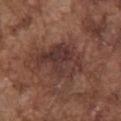The lesion was photographed on a routine skin check and not biopsied; there is no pathology result.
Captured under white-light illumination.
The subject is a male aged around 75.
The lesion is on the chest.
A region of skin cropped from a whole-body photographic capture, roughly 15 mm wide.
Automated image analysis of the tile measured a footprint of about 20 mm², an eccentricity of roughly 0.75, and two-axis asymmetry of about 0.3. The analysis additionally found a lesion color around L≈35 a*≈20 b*≈22 in CIELAB, about 8 CIELAB-L* units darker than the surrounding skin, and a normalized border contrast of about 8.5. The analysis additionally found a nevus-likeness score of about 0/100 and lesion-presence confidence of about 65/100.